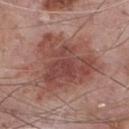  site: front of the torso
  lesion_size:
    long_diameter_mm_approx: 7.5
  patient:
    sex: male
    age_approx: 75
  image:
    source: total-body photography crop
    field_of_view_mm: 15
  automated_metrics:
    area_mm2_approx: 27.0
    eccentricity: 0.5
    shape_asymmetry: 0.5
    cielab_L: 46
    cielab_a: 24
    cielab_b: 24
    vs_skin_darker_L: 11.0
    vs_skin_contrast_norm: 8.0
    color_variation_0_10: 4.5
    nevus_likeness_0_100: 30
    lesion_detection_confidence_0_100: 100
  lighting: white-light